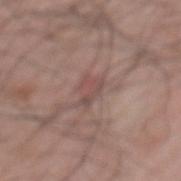Image and clinical context: The lesion is on the mid back. A 15 mm crop from a total-body photograph taken for skin-cancer surveillance. Captured under white-light illumination. A male patient aged 68 to 72. Approximately 3 mm at its widest. An algorithmic analysis of the crop reported a footprint of about 4 mm², a shape eccentricity near 0.8, and two-axis asymmetry of about 0.5. And it measured border irregularity of about 6 on a 0–10 scale, a color-variation rating of about 2/10, and radial color variation of about 1.